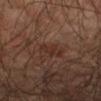Recorded during total-body skin imaging; not selected for excision or biopsy.
A male subject aged approximately 65.
The recorded lesion diameter is about 3 mm.
A roughly 15 mm field-of-view crop from a total-body skin photograph.
On the left forearm.
This is a cross-polarized tile.